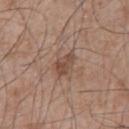The lesion was tiled from a total-body skin photograph and was not biopsied. A 15 mm close-up extracted from a 3D total-body photography capture. Imaged with white-light lighting. On the chest. Automated image analysis of the tile measured an eccentricity of roughly 0.8 and a symmetry-axis asymmetry near 0.3. The software also gave an average lesion color of about L≈48 a*≈18 b*≈26 (CIELAB) and roughly 9 lightness units darker than nearby skin. A male subject, aged around 65. Measured at roughly 3.5 mm in maximum diameter.